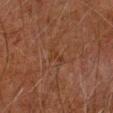Case summary:
- workup · imaged on a skin check; not biopsied
- lighting · cross-polarized
- acquisition · 15 mm crop, total-body photography
- location · the left arm
- lesion size · ≈2.5 mm
- patient · male, in their 60s